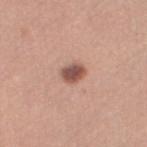Captured during whole-body skin photography for melanoma surveillance; the lesion was not biopsied. A roughly 15 mm field-of-view crop from a total-body skin photograph. The lesion is located on the left thigh. The lesion-visualizer software estimated an area of roughly 4.5 mm² and an outline eccentricity of about 0.7 (0 = round, 1 = elongated). The software also gave a lesion color around L≈52 a*≈22 b*≈25 in CIELAB, about 15 CIELAB-L* units darker than the surrounding skin, and a normalized lesion–skin contrast near 10.5. The software also gave border irregularity of about 1.5 on a 0–10 scale and radial color variation of about 1. The analysis additionally found an automated nevus-likeness rating near 90 out of 100 and lesion-presence confidence of about 100/100. Captured under white-light illumination. A female subject approximately 35 years of age.